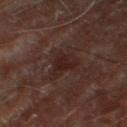Clinical impression:
Imaged during a routine full-body skin examination; the lesion was not biopsied and no histopathology is available.
Image and clinical context:
A close-up tile cropped from a whole-body skin photograph, about 15 mm across. A male patient, in their 70s. Longest diameter approximately 4 mm. On the right thigh.